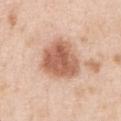The lesion was photographed on a routine skin check and not biopsied; there is no pathology result.
A male subject, roughly 50 years of age.
This image is a 15 mm lesion crop taken from a total-body photograph.
On the chest.